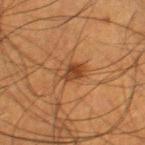Q: Was this lesion biopsied?
A: no biopsy performed (imaged during a skin exam)
Q: Who is the patient?
A: male, about 50 years old
Q: What is the anatomic site?
A: the leg
Q: How was this image acquired?
A: ~15 mm tile from a whole-body skin photo